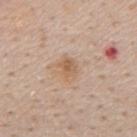| key | value |
|---|---|
| biopsy status | total-body-photography surveillance lesion; no biopsy |
| automated lesion analysis | a lesion area of about 6 mm² and an outline eccentricity of about 0.6 (0 = round, 1 = elongated); a lesion-detection confidence of about 100/100 |
| tile lighting | white-light |
| site | the mid back |
| lesion size | about 3 mm |
| subject | male, in their 50s |
| imaging modality | ~15 mm crop, total-body skin-cancer survey |